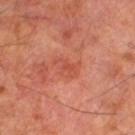Assessment: Part of a total-body skin-imaging series; this lesion was reviewed on a skin check and was not flagged for biopsy.